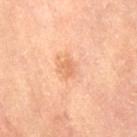This lesion was catalogued during total-body skin photography and was not selected for biopsy. Approximately 2 mm at its widest. A 15 mm close-up tile from a total-body photography series done for melanoma screening. The lesion is on the right thigh. Captured under cross-polarized illumination. A female patient aged 63 to 67.